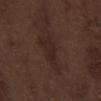A roughly 15 mm field-of-view crop from a total-body skin photograph. Measured at roughly 5.5 mm in maximum diameter. On the abdomen. The patient is a male aged around 70. The tile uses white-light illumination.